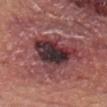workup: no biopsy performed (imaged during a skin exam)
image source: 15 mm crop, total-body photography
location: the chest
subject: male, in their mid-60s